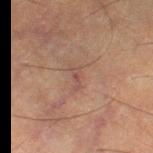<lesion>
  <biopsy_status>not biopsied; imaged during a skin examination</biopsy_status>
  <lighting>cross-polarized</lighting>
  <automated_metrics>
    <area_mm2_approx>3.0</area_mm2_approx>
    <eccentricity>0.9</eccentricity>
    <shape_asymmetry>0.5</shape_asymmetry>
    <cielab_L>39</cielab_L>
    <cielab_a>17</cielab_a>
    <cielab_b>20</cielab_b>
    <vs_skin_darker_L>5.0</vs_skin_darker_L>
    <vs_skin_contrast_norm>5.0</vs_skin_contrast_norm>
    <border_irregularity_0_10>5.5</border_irregularity_0_10>
    <peripheral_color_asymmetry>0.0</peripheral_color_asymmetry>
  </automated_metrics>
  <image>
    <source>total-body photography crop</source>
    <field_of_view_mm>15</field_of_view_mm>
  </image>
  <patient>
    <sex>female</sex>
    <age_approx>60</age_approx>
  </patient>
  <site>right thigh</site>
</lesion>